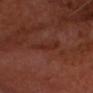No biopsy was performed on this lesion — it was imaged during a full skin examination and was not determined to be concerning.
The subject is a male aged approximately 65.
Cropped from a whole-body photographic skin survey; the tile spans about 15 mm.
Captured under cross-polarized illumination.
The lesion's longest dimension is about 4 mm.